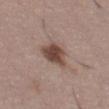| field | value |
|---|---|
| follow-up | imaged on a skin check; not biopsied |
| image source | 15 mm crop, total-body photography |
| site | the leg |
| patient | female, in their 50s |